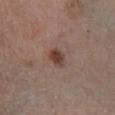workup: catalogued during a skin exam; not biopsied | anatomic site: the right lower leg | diameter: ≈2.5 mm | tile lighting: white-light illumination | patient: male, roughly 55 years of age | image: ~15 mm crop, total-body skin-cancer survey.